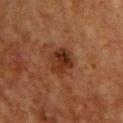Clinical impression: This lesion was catalogued during total-body skin photography and was not selected for biopsy. Background: A lesion tile, about 15 mm wide, cut from a 3D total-body photograph. Imaged with cross-polarized lighting. A male subject in their 60s. On the chest. Automated image analysis of the tile measured an area of roughly 7 mm², a shape eccentricity near 0.55, and two-axis asymmetry of about 0.2. It also reported border irregularity of about 1.5 on a 0–10 scale, internal color variation of about 5.5 on a 0–10 scale, and a peripheral color-asymmetry measure near 2. The analysis additionally found a detector confidence of about 100 out of 100 that the crop contains a lesion.